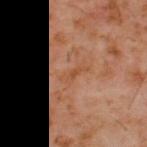- automated metrics · a mean CIELAB color near L≈47 a*≈22 b*≈33, a lesion–skin lightness drop of about 6, and a normalized lesion–skin contrast near 5.5; a border-irregularity index near 5/10 and a peripheral color-asymmetry measure near 0; a classifier nevus-likeness of about 0/100 and lesion-presence confidence of about 100/100
- tile lighting · cross-polarized illumination
- image source · ~15 mm tile from a whole-body skin photo
- patient · male, aged around 60
- location · the upper back
- size · ~3 mm (longest diameter)